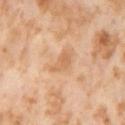<tbp_lesion>
<lesion_size>
  <long_diameter_mm_approx>3.5</long_diameter_mm_approx>
</lesion_size>
<automated_metrics>
  <area_mm2_approx>4.0</area_mm2_approx>
  <eccentricity>0.9</eccentricity>
  <shape_asymmetry>0.45</shape_asymmetry>
</automated_metrics>
<site>leg</site>
<patient>
  <sex>female</sex>
  <age_approx>55</age_approx>
</patient>
<image>
  <source>total-body photography crop</source>
  <field_of_view_mm>15</field_of_view_mm>
</image>
</tbp_lesion>